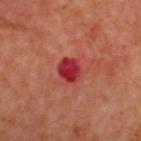workup = no biopsy performed (imaged during a skin exam).